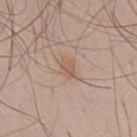– subject — male, in their 40s
– image-analysis metrics — a footprint of about 4.5 mm², a shape eccentricity near 0.75, and a symmetry-axis asymmetry near 0.35; border irregularity of about 3.5 on a 0–10 scale, a within-lesion color-variation index near 2/10, and peripheral color asymmetry of about 0.5; lesion-presence confidence of about 100/100
– size — ≈3 mm
– tile lighting — white-light
– site — the chest
– acquisition — total-body-photography crop, ~15 mm field of view